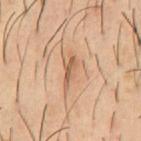Findings:
– biopsy status: imaged on a skin check; not biopsied
– subject: male, roughly 55 years of age
– site: the front of the torso
– lesion diameter: ~4 mm (longest diameter)
– tile lighting: cross-polarized illumination
– automated metrics: a border-irregularity index near 5/10, a color-variation rating of about 3/10, and peripheral color asymmetry of about 1
– acquisition: 15 mm crop, total-body photography